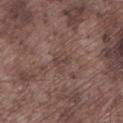Q: Was this lesion biopsied?
A: no biopsy performed (imaged during a skin exam)
Q: How was this image acquired?
A: total-body-photography crop, ~15 mm field of view
Q: Lesion size?
A: ~2.5 mm (longest diameter)
Q: What is the anatomic site?
A: the left lower leg
Q: How was the tile lit?
A: white-light illumination
Q: Patient demographics?
A: male, in their mid- to late 70s